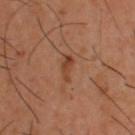Recorded during total-body skin imaging; not selected for excision or biopsy.